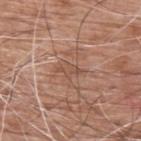Impression: Captured during whole-body skin photography for melanoma surveillance; the lesion was not biopsied. Image and clinical context: A male patient about 60 years old. A close-up tile cropped from a whole-body skin photograph, about 15 mm across. Located on the back. Automated tile analysis of the lesion measured a border-irregularity index near 4/10 and internal color variation of about 0 on a 0–10 scale. It also reported an automated nevus-likeness rating near 0 out of 100 and a detector confidence of about 65 out of 100 that the crop contains a lesion.